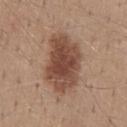Q: What did automated image analysis measure?
A: an area of roughly 24 mm², a shape eccentricity near 0.8, and a shape-asymmetry score of about 0.2 (0 = symmetric); border irregularity of about 2.5 on a 0–10 scale, internal color variation of about 4.5 on a 0–10 scale, and peripheral color asymmetry of about 1; an automated nevus-likeness rating near 95 out of 100
Q: What is the lesion's diameter?
A: ≈7 mm
Q: Lesion location?
A: the mid back
Q: What are the patient's age and sex?
A: male, in their 40s
Q: What kind of image is this?
A: ~15 mm tile from a whole-body skin photo
Q: Illumination type?
A: white-light